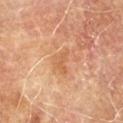<tbp_lesion>
  <biopsy_status>not biopsied; imaged during a skin examination</biopsy_status>
  <lighting>cross-polarized</lighting>
  <image>
    <source>total-body photography crop</source>
    <field_of_view_mm>15</field_of_view_mm>
  </image>
  <automated_metrics>
    <cielab_L>49</cielab_L>
    <cielab_a>19</cielab_a>
    <cielab_b>32</cielab_b>
    <vs_skin_darker_L>6.0</vs_skin_darker_L>
    <vs_skin_contrast_norm>5.0</vs_skin_contrast_norm>
    <peripheral_color_asymmetry>0.5</peripheral_color_asymmetry>
    <nevus_likeness_0_100>0</nevus_likeness_0_100>
    <lesion_detection_confidence_0_100>100</lesion_detection_confidence_0_100>
  </automated_metrics>
  <patient>
    <sex>male</sex>
    <age_approx>70</age_approx>
  </patient>
  <lesion_size>
    <long_diameter_mm_approx>3.5</long_diameter_mm_approx>
  </lesion_size>
  <site>left upper arm</site>
</tbp_lesion>